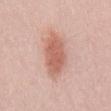Clinical impression:
Captured during whole-body skin photography for melanoma surveillance; the lesion was not biopsied.
Clinical summary:
This image is a 15 mm lesion crop taken from a total-body photograph. Located on the mid back. An algorithmic analysis of the crop reported an average lesion color of about L≈61 a*≈24 b*≈28 (CIELAB), about 12 CIELAB-L* units darker than the surrounding skin, and a lesion-to-skin contrast of about 7.5 (normalized; higher = more distinct). And it measured lesion-presence confidence of about 100/100. Approximately 6 mm at its widest. Captured under white-light illumination. A male patient in their mid-20s.